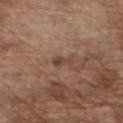Assessment: Part of a total-body skin-imaging series; this lesion was reviewed on a skin check and was not flagged for biopsy. Clinical summary: The lesion is located on the chest. A male subject in their mid-70s. A 15 mm close-up extracted from a 3D total-body photography capture. The recorded lesion diameter is about 2.5 mm. The total-body-photography lesion software estimated an area of roughly 2.5 mm², an eccentricity of roughly 0.9, and two-axis asymmetry of about 0.45. It also reported an average lesion color of about L≈43 a*≈19 b*≈26 (CIELAB), about 8 CIELAB-L* units darker than the surrounding skin, and a normalized border contrast of about 6.5. It also reported a color-variation rating of about 0/10 and a peripheral color-asymmetry measure near 0.